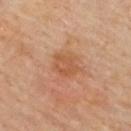notes=imaged on a skin check; not biopsied | body site=the back | image-analysis metrics=a shape eccentricity near 0.6 and two-axis asymmetry of about 0.25; a nevus-likeness score of about 10/100 and a detector confidence of about 100 out of 100 that the crop contains a lesion | patient=female, aged approximately 60 | image source=~15 mm crop, total-body skin-cancer survey.